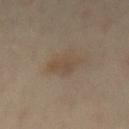workup=imaged on a skin check; not biopsied | patient=male, aged around 55 | image=15 mm crop, total-body photography | lesion diameter=about 3 mm | tile lighting=cross-polarized | body site=the lower back.